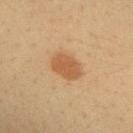Case summary:
- workup · catalogued during a skin exam; not biopsied
- subject · female, approximately 40 years of age
- body site · the left forearm
- illumination · cross-polarized
- image · 15 mm crop, total-body photography
- lesion diameter · about 3.5 mm